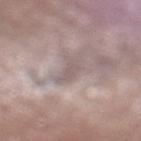patient: male, approximately 75 years of age | diameter: about 3.5 mm | location: the left lower leg | illumination: white-light illumination | automated metrics: a mean CIELAB color near L≈56 a*≈13 b*≈17, a lesion–skin lightness drop of about 8, and a normalized lesion–skin contrast near 5 | imaging modality: total-body-photography crop, ~15 mm field of view | biopsy diagnosis: a seborrheic keratosis — a benign skin lesion.